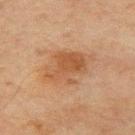A 15 mm crop from a total-body photograph taken for skin-cancer surveillance. The total-body-photography lesion software estimated a lesion color around L≈43 a*≈18 b*≈30 in CIELAB, about 7 CIELAB-L* units darker than the surrounding skin, and a normalized border contrast of about 6.5. It also reported a within-lesion color-variation index near 3.5/10 and a peripheral color-asymmetry measure near 1. The software also gave a classifier nevus-likeness of about 30/100 and a lesion-detection confidence of about 100/100. Approximately 5.5 mm at its widest. Imaged with cross-polarized lighting. Located on the right upper arm. The subject is a male roughly 65 years of age.